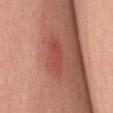<case>
<site>mid back</site>
<patient>
  <sex>female</sex>
  <age_approx>55</age_approx>
</patient>
<lesion_size>
  <long_diameter_mm_approx>5.0</long_diameter_mm_approx>
</lesion_size>
<image>
  <source>total-body photography crop</source>
  <field_of_view_mm>15</field_of_view_mm>
</image>
</case>